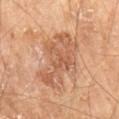Impression:
Part of a total-body skin-imaging series; this lesion was reviewed on a skin check and was not flagged for biopsy.
Image and clinical context:
This is a cross-polarized tile. From the right thigh. The recorded lesion diameter is about 7 mm. Cropped from a whole-body photographic skin survey; the tile spans about 15 mm. An algorithmic analysis of the crop reported a footprint of about 23 mm², a shape eccentricity near 0.75, and a shape-asymmetry score of about 0.35 (0 = symmetric). The software also gave a lesion color around L≈57 a*≈22 b*≈33 in CIELAB, a lesion–skin lightness drop of about 9, and a normalized border contrast of about 6. And it measured a border-irregularity index near 5.5/10, internal color variation of about 4.5 on a 0–10 scale, and a peripheral color-asymmetry measure near 1.5. It also reported a nevus-likeness score of about 0/100 and a lesion-detection confidence of about 100/100. A male patient aged approximately 70.